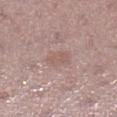Part of a total-body skin-imaging series; this lesion was reviewed on a skin check and was not flagged for biopsy. On the left lower leg. Automated tile analysis of the lesion measured a symmetry-axis asymmetry near 0.3. It also reported a lesion color around L≈57 a*≈18 b*≈22 in CIELAB, roughly 6 lightness units darker than nearby skin, and a lesion-to-skin contrast of about 4.5 (normalized; higher = more distinct). It also reported border irregularity of about 3 on a 0–10 scale, internal color variation of about 1.5 on a 0–10 scale, and radial color variation of about 0.5. The software also gave lesion-presence confidence of about 100/100. The subject is a female aged approximately 55. This image is a 15 mm lesion crop taken from a total-body photograph.